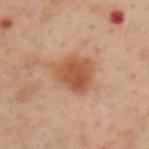biopsy status=total-body-photography surveillance lesion; no biopsy
diameter=≈4.5 mm
illumination=cross-polarized illumination
subject=male, about 55 years old
automated metrics=a footprint of about 13 mm², an eccentricity of roughly 0.6, and a symmetry-axis asymmetry near 0.3; border irregularity of about 2.5 on a 0–10 scale and radial color variation of about 1.5; an automated nevus-likeness rating near 100 out of 100
imaging modality=total-body-photography crop, ~15 mm field of view
site=the upper back